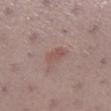  biopsy_status: not biopsied; imaged during a skin examination
  lighting: white-light
  patient:
    sex: female
    age_approx: 55
  image:
    source: total-body photography crop
    field_of_view_mm: 15
  automated_metrics:
    area_mm2_approx: 4.0
    eccentricity: 0.75
    shape_asymmetry: 0.25
    cielab_L: 52
    cielab_a: 19
    cielab_b: 22
    vs_skin_darker_L: 7.0
    vs_skin_contrast_norm: 5.5
    border_irregularity_0_10: 2.5
    color_variation_0_10: 1.5
    peripheral_color_asymmetry: 0.5
    nevus_likeness_0_100: 25
    lesion_detection_confidence_0_100: 100
  site: right lower leg